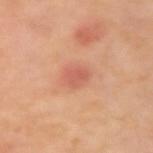Notes:
– follow-up · no biopsy performed (imaged during a skin exam)
– acquisition · ~15 mm tile from a whole-body skin photo
– image-analysis metrics · an area of roughly 4.5 mm², an eccentricity of roughly 0.5, and two-axis asymmetry of about 0.25; a lesion color around L≈60 a*≈29 b*≈32 in CIELAB, roughly 8 lightness units darker than nearby skin, and a lesion-to-skin contrast of about 5.5 (normalized; higher = more distinct); a border-irregularity index near 2/10, internal color variation of about 1.5 on a 0–10 scale, and radial color variation of about 0.5
– lesion size · ≈2.5 mm
– illumination · cross-polarized
– site · the right upper arm
– subject · female, in their mid- to late 50s